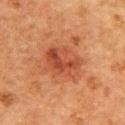<lesion>
  <biopsy_status>not biopsied; imaged during a skin examination</biopsy_status>
  <patient>
    <sex>female</sex>
    <age_approx>60</age_approx>
  </patient>
  <lighting>cross-polarized</lighting>
  <site>upper back</site>
  <automated_metrics>
    <shape_asymmetry>0.2</shape_asymmetry>
    <cielab_L>40</cielab_L>
    <cielab_a>25</cielab_a>
    <cielab_b>31</cielab_b>
    <vs_skin_contrast_norm>6.5</vs_skin_contrast_norm>
    <border_irregularity_0_10>2.0</border_irregularity_0_10>
    <color_variation_0_10>6.0</color_variation_0_10>
    <peripheral_color_asymmetry>2.5</peripheral_color_asymmetry>
    <nevus_likeness_0_100>30</nevus_likeness_0_100>
  </automated_metrics>
  <lesion_size>
    <long_diameter_mm_approx>5.0</long_diameter_mm_approx>
  </lesion_size>
  <image>
    <source>total-body photography crop</source>
    <field_of_view_mm>15</field_of_view_mm>
  </image>
</lesion>